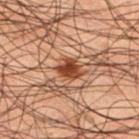Captured during whole-body skin photography for melanoma surveillance; the lesion was not biopsied. A roughly 15 mm field-of-view crop from a total-body skin photograph. The lesion is on the leg. This is a cross-polarized tile. A male subject, approximately 50 years of age. Approximately 2.5 mm at its widest.